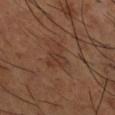No biopsy was performed on this lesion — it was imaged during a full skin examination and was not determined to be concerning. This image is a 15 mm lesion crop taken from a total-body photograph. The lesion is on the right lower leg. The subject is a male aged 63–67.